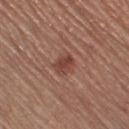Findings:
- follow-up — imaged on a skin check; not biopsied
- lighting — white-light illumination
- site — the right thigh
- subject — female, about 65 years old
- image-analysis metrics — an outline eccentricity of about 0.65 (0 = round, 1 = elongated) and a shape-asymmetry score of about 0.25 (0 = symmetric); a classifier nevus-likeness of about 95/100 and a detector confidence of about 100 out of 100 that the crop contains a lesion
- image source — ~15 mm tile from a whole-body skin photo